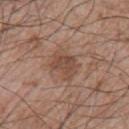Assessment: Captured during whole-body skin photography for melanoma surveillance; the lesion was not biopsied. Clinical summary: Measured at roughly 3.5 mm in maximum diameter. Automated tile analysis of the lesion measured an area of roughly 8.5 mm² and two-axis asymmetry of about 0.3. Cropped from a whole-body photographic skin survey; the tile spans about 15 mm. On the left upper arm. A male patient aged around 65. This is a white-light tile.